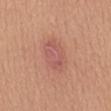automated metrics: a border-irregularity rating of about 2/10 and a peripheral color-asymmetry measure near 1; subject: female, aged 53–57; anatomic site: the mid back; imaging modality: 15 mm crop, total-body photography; diameter: ~5 mm (longest diameter); illumination: white-light.